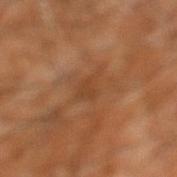This lesion was catalogued during total-body skin photography and was not selected for biopsy.
The lesion is located on the right leg.
This is a cross-polarized tile.
A close-up tile cropped from a whole-body skin photograph, about 15 mm across.
Longest diameter approximately 2.5 mm.
The patient is a male roughly 60 years of age.